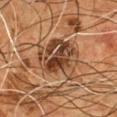No biopsy was performed on this lesion — it was imaged during a full skin examination and was not determined to be concerning.
An algorithmic analysis of the crop reported a footprint of about 21 mm² and an outline eccentricity of about 0.45 (0 = round, 1 = elongated). It also reported a nevus-likeness score of about 20/100.
On the chest.
Measured at roughly 5.5 mm in maximum diameter.
A close-up tile cropped from a whole-body skin photograph, about 15 mm across.
The patient is a male aged around 55.